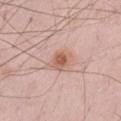patient: male, in their mid-50s | body site: the left thigh | imaging modality: total-body-photography crop, ~15 mm field of view.